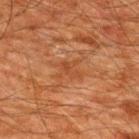workup: total-body-photography surveillance lesion; no biopsy | subject: male, approximately 60 years of age | diameter: about 3 mm | acquisition: ~15 mm tile from a whole-body skin photo | location: the back.